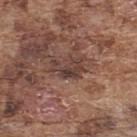| key | value |
|---|---|
| follow-up | total-body-photography surveillance lesion; no biopsy |
| automated metrics | an average lesion color of about L≈39 a*≈18 b*≈22 (CIELAB), roughly 9 lightness units darker than nearby skin, and a normalized lesion–skin contrast near 8; a border-irregularity rating of about 6.5/10, a color-variation rating of about 4.5/10, and a peripheral color-asymmetry measure near 1.5; a classifier nevus-likeness of about 5/100 and a lesion-detection confidence of about 55/100 |
| acquisition | 15 mm crop, total-body photography |
| site | the upper back |
| subject | male, about 75 years old |
| tile lighting | white-light illumination |